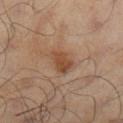A lesion tile, about 15 mm wide, cut from a 3D total-body photograph.
On the left lower leg.
A male subject aged approximately 65.